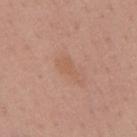Clinical impression: Recorded during total-body skin imaging; not selected for excision or biopsy. Acquisition and patient details: The lesion is on the mid back. Imaged with white-light lighting. Automated image analysis of the tile measured an average lesion color of about L≈58 a*≈21 b*≈31 (CIELAB), a lesion–skin lightness drop of about 5, and a normalized lesion–skin contrast near 4.5. Measured at roughly 4 mm in maximum diameter. A 15 mm close-up extracted from a 3D total-body photography capture. A female patient in their 50s.